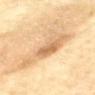notes = imaged on a skin check; not biopsied
body site = the mid back
subject = male, aged 68–72
acquisition = 15 mm crop, total-body photography
lighting = cross-polarized illumination
diameter = ~7.5 mm (longest diameter)